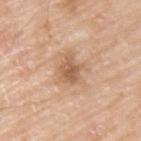  biopsy_status: not biopsied; imaged during a skin examination
  image:
    source: total-body photography crop
    field_of_view_mm: 15
  lighting: white-light
  lesion_size:
    long_diameter_mm_approx: 3.0
  patient:
    sex: male
    age_approx: 65
  site: upper back